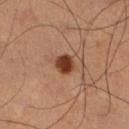The lesion was photographed on a routine skin check and not biopsied; there is no pathology result.
Captured under cross-polarized illumination.
Automated image analysis of the tile measured a lesion area of about 5 mm², an outline eccentricity of about 0.5 (0 = round, 1 = elongated), and a shape-asymmetry score of about 0.25 (0 = symmetric). The software also gave a border-irregularity rating of about 2/10, internal color variation of about 3 on a 0–10 scale, and peripheral color asymmetry of about 1. And it measured a lesion-detection confidence of about 100/100.
The lesion's longest dimension is about 2.5 mm.
The subject is a male aged 58–62.
On the right lower leg.
Cropped from a total-body skin-imaging series; the visible field is about 15 mm.